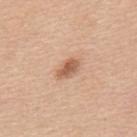Clinical impression:
No biopsy was performed on this lesion — it was imaged during a full skin examination and was not determined to be concerning.
Background:
Longest diameter approximately 3 mm. Automated tile analysis of the lesion measured a nevus-likeness score of about 90/100 and a lesion-detection confidence of about 100/100. The lesion is on the upper back. The patient is a male in their 50s. A roughly 15 mm field-of-view crop from a total-body skin photograph. Imaged with white-light lighting.